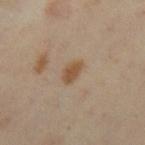* workup: imaged on a skin check; not biopsied
* image: total-body-photography crop, ~15 mm field of view
* anatomic site: the right thigh
* size: ≈3 mm
* lighting: cross-polarized illumination
* patient: female, in their mid- to late 30s